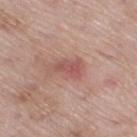| field | value |
|---|---|
| follow-up | catalogued during a skin exam; not biopsied |
| diameter | about 4 mm |
| subject | male, in their 70s |
| image | ~15 mm tile from a whole-body skin photo |
| location | the right thigh |
| lighting | white-light |
| automated lesion analysis | an outline eccentricity of about 0.85 (0 = round, 1 = elongated) and a shape-asymmetry score of about 0.4 (0 = symmetric); an average lesion color of about L≈54 a*≈25 b*≈24 (CIELAB), a lesion–skin lightness drop of about 9, and a normalized border contrast of about 6; an automated nevus-likeness rating near 10 out of 100 and a detector confidence of about 100 out of 100 that the crop contains a lesion |